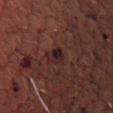notes: imaged on a skin check; not biopsied
imaging modality: 15 mm crop, total-body photography
subject: male, aged 48–52
illumination: cross-polarized illumination
body site: the left thigh